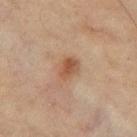Q: Was a biopsy performed?
A: total-body-photography surveillance lesion; no biopsy
Q: Lesion location?
A: the leg
Q: What is the imaging modality?
A: ~15 mm crop, total-body skin-cancer survey
Q: Patient demographics?
A: female, aged 53 to 57
Q: What did automated image analysis measure?
A: a footprint of about 5 mm², an eccentricity of roughly 0.7, and two-axis asymmetry of about 0.2; a lesion–skin lightness drop of about 9; a border-irregularity rating of about 1.5/10 and internal color variation of about 3 on a 0–10 scale; an automated nevus-likeness rating near 75 out of 100 and a lesion-detection confidence of about 100/100